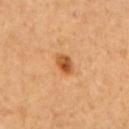Notes:
• follow-up: no biopsy performed (imaged during a skin exam)
• anatomic site: the upper back
• subject: female, aged around 65
• image source: ~15 mm tile from a whole-body skin photo
• tile lighting: cross-polarized illumination
• image-analysis metrics: a lesion color around L≈54 a*≈26 b*≈43 in CIELAB, about 13 CIELAB-L* units darker than the surrounding skin, and a normalized border contrast of about 9; a border-irregularity index near 1.5/10, internal color variation of about 6.5 on a 0–10 scale, and peripheral color asymmetry of about 2.5; an automated nevus-likeness rating near 95 out of 100 and lesion-presence confidence of about 100/100
• lesion size: ≈3 mm